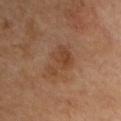Captured during whole-body skin photography for melanoma surveillance; the lesion was not biopsied.
A male patient roughly 65 years of age.
Measured at roughly 4 mm in maximum diameter.
Cropped from a whole-body photographic skin survey; the tile spans about 15 mm.
The lesion-visualizer software estimated an area of roughly 8 mm², an eccentricity of roughly 0.75, and two-axis asymmetry of about 0.45. And it measured a lesion–skin lightness drop of about 7.
The lesion is on the chest.
The tile uses cross-polarized illumination.